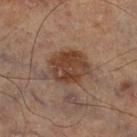Clinical impression: Captured during whole-body skin photography for melanoma surveillance; the lesion was not biopsied. Clinical summary: Longest diameter approximately 6 mm. Automated tile analysis of the lesion measured an area of roughly 17 mm², a shape eccentricity near 0.7, and a shape-asymmetry score of about 0.2 (0 = symmetric). It also reported about 10 CIELAB-L* units darker than the surrounding skin and a lesion-to-skin contrast of about 8.5 (normalized; higher = more distinct). A lesion tile, about 15 mm wide, cut from a 3D total-body photograph. The lesion is on the right thigh.